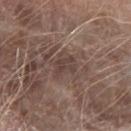{
  "biopsy_status": "not biopsied; imaged during a skin examination",
  "site": "left forearm",
  "image": {
    "source": "total-body photography crop",
    "field_of_view_mm": 15
  },
  "automated_metrics": {
    "cielab_L": 40,
    "cielab_a": 15,
    "cielab_b": 20,
    "vs_skin_darker_L": 7.0,
    "vs_skin_contrast_norm": 6.0,
    "border_irregularity_0_10": 5.0,
    "color_variation_0_10": 1.0,
    "peripheral_color_asymmetry": 0.5
  },
  "lesion_size": {
    "long_diameter_mm_approx": 2.5
  },
  "patient": {
    "sex": "male",
    "age_approx": 80
  },
  "lighting": "white-light"
}